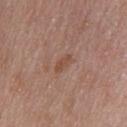No biopsy was performed on this lesion — it was imaged during a full skin examination and was not determined to be concerning. Cropped from a total-body skin-imaging series; the visible field is about 15 mm. A male patient roughly 50 years of age. Located on the upper back. Automated tile analysis of the lesion measured an outline eccentricity of about 0.9 (0 = round, 1 = elongated) and a shape-asymmetry score of about 0.3 (0 = symmetric). It also reported a within-lesion color-variation index near 0/10 and peripheral color asymmetry of about 0. The analysis additionally found a nevus-likeness score of about 0/100 and lesion-presence confidence of about 100/100.